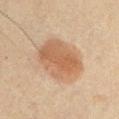Q: Is there a histopathology result?
A: imaged on a skin check; not biopsied